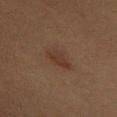follow-up: total-body-photography surveillance lesion; no biopsy | subject: female, aged 53 to 57 | tile lighting: cross-polarized | automated metrics: a lesion area of about 5.5 mm², an eccentricity of roughly 0.85, and a shape-asymmetry score of about 0.3 (0 = symmetric); a border-irregularity index near 3.5/10, a color-variation rating of about 1.5/10, and radial color variation of about 0.5 | body site: the chest | image source: ~15 mm tile from a whole-body skin photo.